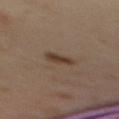No biopsy was performed on this lesion — it was imaged during a full skin examination and was not determined to be concerning. Measured at roughly 3 mm in maximum diameter. From the upper back. A female patient aged 38–42. Captured under cross-polarized illumination. A 15 mm close-up extracted from a 3D total-body photography capture.